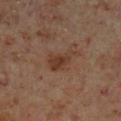workup = total-body-photography surveillance lesion; no biopsy | image source = total-body-photography crop, ~15 mm field of view | anatomic site = the left lower leg | subject = male, about 60 years old | lesion diameter = ~4 mm (longest diameter).